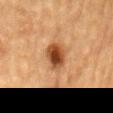{
  "biopsy_status": "not biopsied; imaged during a skin examination",
  "site": "back",
  "patient": {
    "sex": "male",
    "age_approx": "85+"
  },
  "lighting": "cross-polarized",
  "automated_metrics": {
    "nevus_likeness_0_100": 100
  },
  "lesion_size": {
    "long_diameter_mm_approx": 3.5
  },
  "image": {
    "source": "total-body photography crop",
    "field_of_view_mm": 15
  }
}